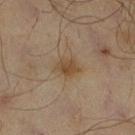Assessment:
The lesion was tiled from a total-body skin photograph and was not biopsied.
Clinical summary:
On the leg. The patient is a male approximately 65 years of age. Automated tile analysis of the lesion measured an area of roughly 3.5 mm² and an eccentricity of roughly 0.75. And it measured an average lesion color of about L≈42 a*≈14 b*≈30 (CIELAB) and a lesion–skin lightness drop of about 8. The software also gave a border-irregularity index near 2/10, a within-lesion color-variation index near 1.5/10, and a peripheral color-asymmetry measure near 0.5. The software also gave an automated nevus-likeness rating near 75 out of 100 and a lesion-detection confidence of about 100/100. The recorded lesion diameter is about 2.5 mm. A 15 mm close-up extracted from a 3D total-body photography capture.